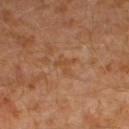biopsy status — catalogued during a skin exam; not biopsied | subject — male, aged 28–32 | acquisition — 15 mm crop, total-body photography | size — ≈2.5 mm | image-analysis metrics — a mean CIELAB color near L≈47 a*≈21 b*≈34 and about 5 CIELAB-L* units darker than the surrounding skin; a classifier nevus-likeness of about 0/100 and a lesion-detection confidence of about 100/100 | illumination — cross-polarized illumination | body site — the left lower leg.